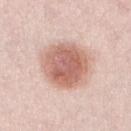{"biopsy_status": "not biopsied; imaged during a skin examination", "patient": {"sex": "female", "age_approx": 30}, "lesion_size": {"long_diameter_mm_approx": 6.0}, "image": {"source": "total-body photography crop", "field_of_view_mm": 15}, "site": "leg", "lighting": "white-light"}